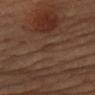follow-up = catalogued during a skin exam; not biopsied | patient = female, aged approximately 65 | image source = 15 mm crop, total-body photography | tile lighting = cross-polarized | anatomic site = the left upper arm.